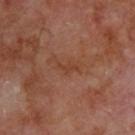{
  "lighting": "cross-polarized",
  "lesion_size": {
    "long_diameter_mm_approx": 2.5
  },
  "patient": {
    "sex": "male",
    "age_approx": 70
  },
  "image": {
    "source": "total-body photography crop",
    "field_of_view_mm": 15
  },
  "site": "upper back"
}